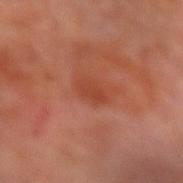Q: Is there a histopathology result?
A: imaged on a skin check; not biopsied
Q: Who is the patient?
A: male, aged around 70
Q: Lesion size?
A: ≈3 mm
Q: What is the imaging modality?
A: ~15 mm tile from a whole-body skin photo
Q: What lighting was used for the tile?
A: cross-polarized illumination
Q: Lesion location?
A: the right forearm